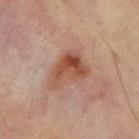{"biopsy_status": "not biopsied; imaged during a skin examination", "lighting": "cross-polarized", "patient": {"sex": "female", "age_approx": 55}, "lesion_size": {"long_diameter_mm_approx": 4.5}, "site": "left thigh", "automated_metrics": {"cielab_L": 43, "cielab_a": 21, "cielab_b": 27, "vs_skin_darker_L": 10.0}, "image": {"source": "total-body photography crop", "field_of_view_mm": 15}}